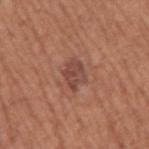* image: total-body-photography crop, ~15 mm field of view
* patient: male, aged around 75
* size: ≈3.5 mm
* location: the right upper arm
* lighting: white-light